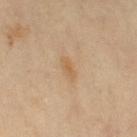Q: Is there a histopathology result?
A: total-body-photography surveillance lesion; no biopsy
Q: Lesion size?
A: about 3 mm
Q: Lesion location?
A: the right thigh
Q: Who is the patient?
A: female, aged 73–77
Q: How was this image acquired?
A: total-body-photography crop, ~15 mm field of view
Q: What did automated image analysis measure?
A: a lesion–skin lightness drop of about 6 and a lesion-to-skin contrast of about 5.5 (normalized; higher = more distinct); a nevus-likeness score of about 0/100 and a lesion-detection confidence of about 100/100